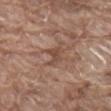No biopsy was performed on this lesion — it was imaged during a full skin examination and was not determined to be concerning.
The patient is a male about 80 years old.
A 15 mm close-up tile from a total-body photography series done for melanoma screening.
An algorithmic analysis of the crop reported a lesion color around L≈48 a*≈19 b*≈27 in CIELAB, roughly 8 lightness units darker than nearby skin, and a normalized border contrast of about 6. It also reported a border-irregularity index near 4.5/10 and internal color variation of about 3 on a 0–10 scale.
Longest diameter approximately 3 mm.
From the mid back.